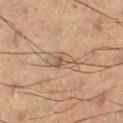Findings:
- follow-up: no biopsy performed (imaged during a skin exam)
- size: ≈4 mm
- site: the right lower leg
- lighting: cross-polarized illumination
- image source: 15 mm crop, total-body photography
- patient: male, approximately 55 years of age